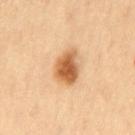Context:
A female subject, about 45 years old. A region of skin cropped from a whole-body photographic capture, roughly 15 mm wide. The lesion is on the left thigh. Captured under cross-polarized illumination. An algorithmic analysis of the crop reported a footprint of about 10 mm², an eccentricity of roughly 0.75, and a symmetry-axis asymmetry near 0.2.